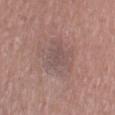Case summary:
- biopsy status: imaged on a skin check; not biopsied
- tile lighting: white-light
- body site: the right thigh
- image: 15 mm crop, total-body photography
- subject: female, roughly 55 years of age
- automated lesion analysis: an average lesion color of about L≈52 a*≈17 b*≈20 (CIELAB) and a normalized lesion–skin contrast near 4.5; a classifier nevus-likeness of about 0/100 and a lesion-detection confidence of about 95/100
- lesion diameter: ~5 mm (longest diameter)